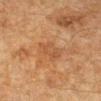<tbp_lesion>
<biopsy_status>not biopsied; imaged during a skin examination</biopsy_status>
<patient>
  <sex>female</sex>
  <age_approx>55</age_approx>
</patient>
<site>left forearm</site>
<lesion_size>
  <long_diameter_mm_approx>4.0</long_diameter_mm_approx>
</lesion_size>
<lighting>cross-polarized</lighting>
<image>
  <source>total-body photography crop</source>
  <field_of_view_mm>15</field_of_view_mm>
</image>
<automated_metrics>
  <eccentricity>0.85</eccentricity>
  <shape_asymmetry>0.3</shape_asymmetry>
  <color_variation_0_10>2.0</color_variation_0_10>
  <peripheral_color_asymmetry>1.0</peripheral_color_asymmetry>
  <nevus_likeness_0_100>0</nevus_likeness_0_100>
  <lesion_detection_confidence_0_100>100</lesion_detection_confidence_0_100>
</automated_metrics>
</tbp_lesion>